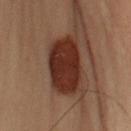– follow-up — catalogued during a skin exam; not biopsied
– lesion size — ~6 mm (longest diameter)
– lighting — cross-polarized illumination
– subject — male, in their mid- to late 50s
– body site — the arm
– imaging modality — 15 mm crop, total-body photography